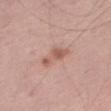{"biopsy_status": "not biopsied; imaged during a skin examination", "lesion_size": {"long_diameter_mm_approx": 4.0}, "site": "right thigh", "automated_metrics": {"area_mm2_approx": 5.0, "shape_asymmetry": 0.4, "vs_skin_contrast_norm": 7.0, "border_irregularity_0_10": 5.0, "color_variation_0_10": 1.5, "peripheral_color_asymmetry": 0.5, "nevus_likeness_0_100": 5, "lesion_detection_confidence_0_100": 100}, "patient": {"sex": "male", "age_approx": 60}, "lighting": "white-light", "image": {"source": "total-body photography crop", "field_of_view_mm": 15}}